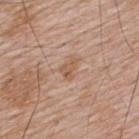Q: Was a biopsy performed?
A: imaged on a skin check; not biopsied
Q: What is the imaging modality?
A: ~15 mm tile from a whole-body skin photo
Q: Patient demographics?
A: male, in their mid- to late 60s
Q: What is the anatomic site?
A: the upper back
Q: Illumination type?
A: white-light
Q: Automated lesion metrics?
A: an average lesion color of about L≈56 a*≈20 b*≈31 (CIELAB), roughly 8 lightness units darker than nearby skin, and a normalized lesion–skin contrast near 6.5
Q: What is the lesion's diameter?
A: ~2.5 mm (longest diameter)